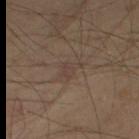Q: Was this lesion biopsied?
A: imaged on a skin check; not biopsied
Q: What lighting was used for the tile?
A: cross-polarized illumination
Q: How was this image acquired?
A: ~15 mm crop, total-body skin-cancer survey
Q: Patient demographics?
A: male, roughly 40 years of age
Q: Lesion location?
A: the right thigh